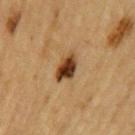Located on the arm. The subject is a male aged 83 or older. A 15 mm close-up extracted from a 3D total-body photography capture. Longest diameter approximately 3.5 mm. Captured under cross-polarized illumination.